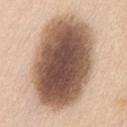Assessment: This lesion was catalogued during total-body skin photography and was not selected for biopsy. Clinical summary: A roughly 15 mm field-of-view crop from a total-body skin photograph. The lesion is located on the front of the torso. A female subject aged approximately 55. About 11.5 mm across. An algorithmic analysis of the crop reported about 24 CIELAB-L* units darker than the surrounding skin and a normalized border contrast of about 14. It also reported a within-lesion color-variation index near 9.5/10. The software also gave a nevus-likeness score of about 90/100 and a detector confidence of about 100 out of 100 that the crop contains a lesion. Imaged with white-light lighting.